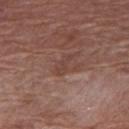Cropped from a whole-body photographic skin survey; the tile spans about 15 mm. The patient is a female roughly 70 years of age. On the right upper arm.